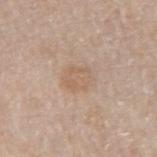Imaged during a routine full-body skin examination; the lesion was not biopsied and no histopathology is available. The tile uses white-light illumination. The lesion-visualizer software estimated a border-irregularity index near 1.5/10 and a within-lesion color-variation index near 2/10. The lesion is on the left forearm. A 15 mm crop from a total-body photograph taken for skin-cancer surveillance. A female subject aged around 45.